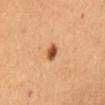workup: catalogued during a skin exam; not biopsied
illumination: cross-polarized
image source: total-body-photography crop, ~15 mm field of view
lesion diameter: ~2.5 mm (longest diameter)
anatomic site: the front of the torso
automated lesion analysis: an area of roughly 3.5 mm², an eccentricity of roughly 0.7, and a symmetry-axis asymmetry near 0.15; an average lesion color of about L≈54 a*≈24 b*≈38 (CIELAB) and about 16 CIELAB-L* units darker than the surrounding skin; internal color variation of about 5.5 on a 0–10 scale and radial color variation of about 1.5
patient: female